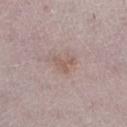anatomic site: the left lower leg | lesion diameter: about 3 mm | image source: total-body-photography crop, ~15 mm field of view | automated metrics: an average lesion color of about L≈56 a*≈17 b*≈23 (CIELAB) and roughly 7 lightness units darker than nearby skin; a border-irregularity rating of about 6.5/10 and internal color variation of about 1 on a 0–10 scale; lesion-presence confidence of about 100/100 | lighting: white-light illumination | patient: female, aged 48–52.